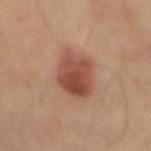Clinical impression:
Captured during whole-body skin photography for melanoma surveillance; the lesion was not biopsied.
Image and clinical context:
The total-body-photography lesion software estimated a lesion color around L≈49 a*≈24 b*≈29 in CIELAB, about 13 CIELAB-L* units darker than the surrounding skin, and a normalized lesion–skin contrast near 9. And it measured internal color variation of about 6 on a 0–10 scale and radial color variation of about 2. A 15 mm close-up tile from a total-body photography series done for melanoma screening. Measured at roughly 5 mm in maximum diameter. On the mid back. This is a cross-polarized tile.